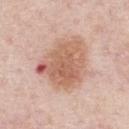subject: male, about 60 years old | image-analysis metrics: an area of roughly 25 mm², an eccentricity of roughly 0.55, and a shape-asymmetry score of about 0.3 (0 = symmetric); a border-irregularity index near 3.5/10 and radial color variation of about 2.5 | image source: ~15 mm crop, total-body skin-cancer survey | body site: the chest | diameter: ≈6.5 mm | tile lighting: white-light illumination.